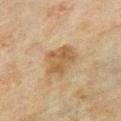Findings:
– biopsy status — no biopsy performed (imaged during a skin exam)
– body site — the left upper arm
– lesion diameter — ≈4.5 mm
– automated lesion analysis — a lesion area of about 12 mm², a shape eccentricity near 0.5, and two-axis asymmetry of about 0.3; a lesion color around L≈47 a*≈14 b*≈31 in CIELAB, about 8 CIELAB-L* units darker than the surrounding skin, and a lesion-to-skin contrast of about 7 (normalized; higher = more distinct)
– subject — male, in their 70s
– image — total-body-photography crop, ~15 mm field of view